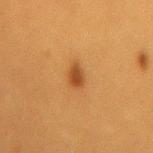Located on the lower back. The tile uses cross-polarized illumination. About 3 mm across. The patient is a female aged 38–42. A region of skin cropped from a whole-body photographic capture, roughly 15 mm wide.